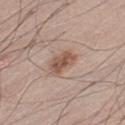Captured during whole-body skin photography for melanoma surveillance; the lesion was not biopsied.
A roughly 15 mm field-of-view crop from a total-body skin photograph.
On the leg.
A male subject in their 40s.